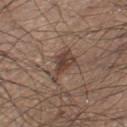Impression: Part of a total-body skin-imaging series; this lesion was reviewed on a skin check and was not flagged for biopsy. Acquisition and patient details: A male patient aged around 45. The total-body-photography lesion software estimated an average lesion color of about L≈41 a*≈16 b*≈23 (CIELAB) and a normalized border contrast of about 8. The software also gave a detector confidence of about 100 out of 100 that the crop contains a lesion. From the right lower leg. A lesion tile, about 15 mm wide, cut from a 3D total-body photograph.